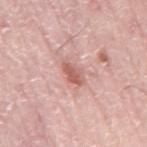notes=catalogued during a skin exam; not biopsied
size=≈3 mm
automated metrics=a footprint of about 4.5 mm², an eccentricity of roughly 0.8, and two-axis asymmetry of about 0.25; a lesion color around L≈60 a*≈25 b*≈26 in CIELAB and roughly 11 lightness units darker than nearby skin; a border-irregularity index near 2.5/10, a color-variation rating of about 3.5/10, and radial color variation of about 1; an automated nevus-likeness rating near 0 out of 100
illumination=white-light
location=the mid back
imaging modality=~15 mm crop, total-body skin-cancer survey
subject=male, about 75 years old